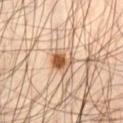This lesion was catalogued during total-body skin photography and was not selected for biopsy. The recorded lesion diameter is about 3 mm. The total-body-photography lesion software estimated a lesion area of about 4.5 mm². Imaged with cross-polarized lighting. A 15 mm close-up tile from a total-body photography series done for melanoma screening. A male patient roughly 45 years of age. Located on the left thigh.